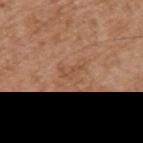Assessment: No biopsy was performed on this lesion — it was imaged during a full skin examination and was not determined to be concerning. Context: The recorded lesion diameter is about 2.5 mm. The lesion is on the left upper arm. A male patient aged 73–77. The lesion-visualizer software estimated an average lesion color of about L≈50 a*≈21 b*≈33 (CIELAB), roughly 6 lightness units darker than nearby skin, and a normalized border contrast of about 4.5. A lesion tile, about 15 mm wide, cut from a 3D total-body photograph.